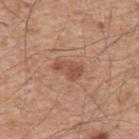biopsy_status: not biopsied; imaged during a skin examination
patient:
  sex: male
  age_approx: 55
site: back
image:
  source: total-body photography crop
  field_of_view_mm: 15
lighting: white-light
automated_metrics:
  cielab_L: 51
  cielab_a: 22
  cielab_b: 31
  vs_skin_contrast_norm: 6.5